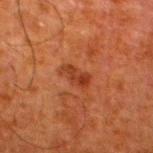Impression:
Part of a total-body skin-imaging series; this lesion was reviewed on a skin check and was not flagged for biopsy.
Acquisition and patient details:
From the leg. The patient is a male aged around 80. A 15 mm close-up tile from a total-body photography series done for melanoma screening. The total-body-photography lesion software estimated a lesion area of about 5 mm², an outline eccentricity of about 0.85 (0 = round, 1 = elongated), and a shape-asymmetry score of about 0.25 (0 = symmetric). The software also gave a mean CIELAB color near L≈32 a*≈25 b*≈31, about 7 CIELAB-L* units darker than the surrounding skin, and a normalized border contrast of about 6.5. The lesion's longest dimension is about 3.5 mm.